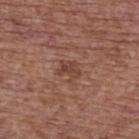notes: no biopsy performed (imaged during a skin exam)
image source: ~15 mm tile from a whole-body skin photo
diameter: ≈2.5 mm
tile lighting: white-light illumination
subject: female, aged 63 to 67
anatomic site: the upper back